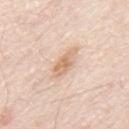Case summary:
• notes — total-body-photography surveillance lesion; no biopsy
• imaging modality — 15 mm crop, total-body photography
• tile lighting — white-light
• site — the mid back
• TBP lesion metrics — roughly 10 lightness units darker than nearby skin and a lesion-to-skin contrast of about 7 (normalized; higher = more distinct); a border-irregularity index near 2.5/10, internal color variation of about 3 on a 0–10 scale, and radial color variation of about 1; lesion-presence confidence of about 100/100
• subject — male, roughly 80 years of age
• lesion diameter — ≈4 mm